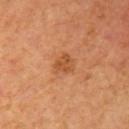Recorded during total-body skin imaging; not selected for excision or biopsy. On the right upper arm. Longest diameter approximately 2.5 mm. A female patient aged 38 to 42. A 15 mm crop from a total-body photograph taken for skin-cancer surveillance. Automated image analysis of the tile measured an area of roughly 4.5 mm², a shape eccentricity near 0.45, and a symmetry-axis asymmetry near 0.3. The software also gave an average lesion color of about L≈52 a*≈27 b*≈40 (CIELAB), a lesion–skin lightness drop of about 8, and a normalized border contrast of about 6.5. And it measured a border-irregularity rating of about 2.5/10 and radial color variation of about 1.